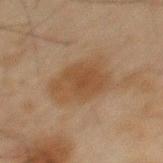notes: catalogued during a skin exam; not biopsied | diameter: ~5.5 mm (longest diameter) | patient: male, about 45 years old | image source: ~15 mm tile from a whole-body skin photo | automated metrics: a footprint of about 18 mm², an eccentricity of roughly 0.7, and a shape-asymmetry score of about 0.2 (0 = symmetric); a border-irregularity rating of about 2.5/10, internal color variation of about 3 on a 0–10 scale, and peripheral color asymmetry of about 1 | body site: the mid back.